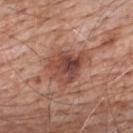| key | value |
|---|---|
| notes | catalogued during a skin exam; not biopsied |
| patient | male, in their 80s |
| tile lighting | white-light |
| site | the upper back |
| diameter | ~5 mm (longest diameter) |
| imaging modality | ~15 mm crop, total-body skin-cancer survey |